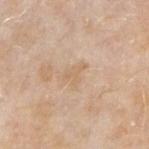biopsy status: no biopsy performed (imaged during a skin exam)
automated lesion analysis: a shape eccentricity near 0.8 and a shape-asymmetry score of about 0.6 (0 = symmetric); a border-irregularity index near 7/10, internal color variation of about 0 on a 0–10 scale, and peripheral color asymmetry of about 0; a nevus-likeness score of about 0/100 and a lesion-detection confidence of about 100/100
patient: male, about 75 years old
imaging modality: 15 mm crop, total-body photography
lesion size: ≈3 mm
location: the right forearm